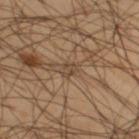Clinical impression: The lesion was tiled from a total-body skin photograph and was not biopsied. Background: Automated tile analysis of the lesion measured a lesion area of about 2 mm² and an eccentricity of roughly 0.25. The analysis additionally found a mean CIELAB color near L≈43 a*≈15 b*≈28, roughly 7 lightness units darker than nearby skin, and a normalized lesion–skin contrast near 6. And it measured an automated nevus-likeness rating near 0 out of 100. A male patient aged around 45. Measured at roughly 1.5 mm in maximum diameter. The lesion is located on the leg. Captured under cross-polarized illumination. A 15 mm close-up extracted from a 3D total-body photography capture.